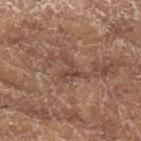About 3.5 mm across. Located on the left forearm. The lesion-visualizer software estimated a lesion area of about 4 mm², an eccentricity of roughly 0.65, and two-axis asymmetry of about 0.7. A 15 mm close-up extracted from a 3D total-body photography capture. A female patient, in their mid-70s.